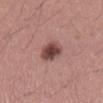Clinical impression:
No biopsy was performed on this lesion — it was imaged during a full skin examination and was not determined to be concerning.
Image and clinical context:
A close-up tile cropped from a whole-body skin photograph, about 15 mm across. Measured at roughly 3.5 mm in maximum diameter. The lesion is located on the mid back. A male patient in their mid- to late 30s. An algorithmic analysis of the crop reported a lesion area of about 7 mm² and an eccentricity of roughly 0.7. And it measured an automated nevus-likeness rating near 90 out of 100 and a lesion-detection confidence of about 100/100.